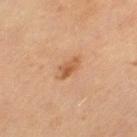<tbp_lesion>
  <biopsy_status>not biopsied; imaged during a skin examination</biopsy_status>
  <site>left thigh</site>
  <lighting>cross-polarized</lighting>
  <automated_metrics>
    <area_mm2_approx>3.0</area_mm2_approx>
    <eccentricity>0.85</eccentricity>
    <shape_asymmetry>0.3</shape_asymmetry>
    <border_irregularity_0_10>3.0</border_irregularity_0_10>
    <peripheral_color_asymmetry>1.0</peripheral_color_asymmetry>
    <nevus_likeness_0_100>60</nevus_likeness_0_100>
    <lesion_detection_confidence_0_100>100</lesion_detection_confidence_0_100>
  </automated_metrics>
  <patient>
    <sex>female</sex>
    <age_approx>70</age_approx>
  </patient>
  <lesion_size>
    <long_diameter_mm_approx>3.0</long_diameter_mm_approx>
  </lesion_size>
  <image>
    <source>total-body photography crop</source>
    <field_of_view_mm>15</field_of_view_mm>
  </image>
</tbp_lesion>